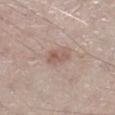Clinical impression: Recorded during total-body skin imaging; not selected for excision or biopsy. Background: A 15 mm crop from a total-body photograph taken for skin-cancer surveillance. A male subject, about 60 years old. The lesion is located on the right lower leg.